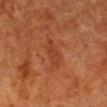Clinical impression:
This lesion was catalogued during total-body skin photography and was not selected for biopsy.
Context:
The patient is a male approximately 80 years of age. Cropped from a whole-body photographic skin survey; the tile spans about 15 mm. On the leg.